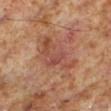From the left lower leg. The patient is a male aged around 60. A 15 mm close-up extracted from a 3D total-body photography capture.